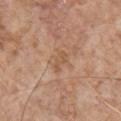biopsy status: imaged on a skin check; not biopsied | anatomic site: the chest | acquisition: total-body-photography crop, ~15 mm field of view | lighting: white-light | automated lesion analysis: an eccentricity of roughly 0.9; a lesion color around L≈54 a*≈20 b*≈33 in CIELAB, roughly 7 lightness units darker than nearby skin, and a normalized lesion–skin contrast near 5; a within-lesion color-variation index near 0/10 and peripheral color asymmetry of about 0 | subject: male, aged 53 to 57 | diameter: ≈2.5 mm.